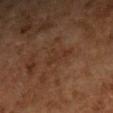  biopsy_status: not biopsied; imaged during a skin examination
  image:
    source: total-body photography crop
    field_of_view_mm: 15
  site: arm
  lighting: cross-polarized
  patient:
    sex: female
    age_approx: 60